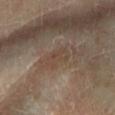Clinical impression:
Imaged during a routine full-body skin examination; the lesion was not biopsied and no histopathology is available.
Clinical summary:
The lesion is on the left lower leg. The lesion-visualizer software estimated a mean CIELAB color near L≈42 a*≈14 b*≈24 and roughly 5 lightness units darker than nearby skin. The software also gave a nevus-likeness score of about 0/100. A close-up tile cropped from a whole-body skin photograph, about 15 mm across. Approximately 3.5 mm at its widest. The tile uses cross-polarized illumination. A male subject, aged 68–72.